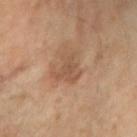{"biopsy_status": "not biopsied; imaged during a skin examination", "image": {"source": "total-body photography crop", "field_of_view_mm": 15}, "lighting": "cross-polarized", "lesion_size": {"long_diameter_mm_approx": 4.0}, "site": "right forearm", "patient": {"sex": "female", "age_approx": 70}}